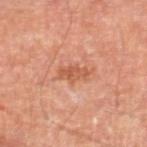Impression: Recorded during total-body skin imaging; not selected for excision or biopsy. Image and clinical context: The lesion is located on the leg. This image is a 15 mm lesion crop taken from a total-body photograph. Measured at roughly 3.5 mm in maximum diameter. A male patient aged around 70.